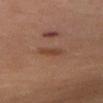TBP lesion metrics: a mean CIELAB color near L≈41 a*≈20 b*≈29, roughly 7 lightness units darker than nearby skin, and a normalized lesion–skin contrast near 6.5; a border-irregularity rating of about 2.5/10, internal color variation of about 2.5 on a 0–10 scale, and peripheral color asymmetry of about 1; an automated nevus-likeness rating near 35 out of 100 and lesion-presence confidence of about 100/100
site: the upper back
diameter: ≈2.5 mm
acquisition: 15 mm crop, total-body photography
tile lighting: cross-polarized
patient: female, aged approximately 50